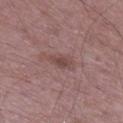This lesion was catalogued during total-body skin photography and was not selected for biopsy. A male patient, about 50 years old. The tile uses white-light illumination. The recorded lesion diameter is about 3 mm. The lesion is located on the right lower leg. A region of skin cropped from a whole-body photographic capture, roughly 15 mm wide.